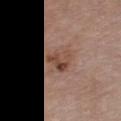Part of a total-body skin-imaging series; this lesion was reviewed on a skin check and was not flagged for biopsy. A male patient aged 73–77. A roughly 15 mm field-of-view crop from a total-body skin photograph. The lesion is on the chest.